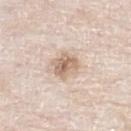The lesion was tiled from a total-body skin photograph and was not biopsied.
The lesion is on the left lower leg.
Cropped from a total-body skin-imaging series; the visible field is about 15 mm.
A male patient, aged 78 to 82.
Automated image analysis of the tile measured a border-irregularity rating of about 2/10 and a color-variation rating of about 5.5/10.